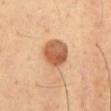{
  "lesion_size": {
    "long_diameter_mm_approx": 4.5
  },
  "site": "abdomen",
  "image": {
    "source": "total-body photography crop",
    "field_of_view_mm": 15
  },
  "patient": {
    "sex": "male",
    "age_approx": 65
  },
  "lighting": "cross-polarized"
}